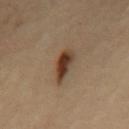Imaged with cross-polarized lighting. Cropped from a whole-body photographic skin survey; the tile spans about 15 mm. The lesion's longest dimension is about 4.5 mm. From the upper back. A female patient, aged around 60.